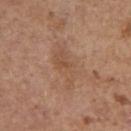Impression:
The lesion was tiled from a total-body skin photograph and was not biopsied.
Clinical summary:
The patient is a female aged approximately 65. Imaged with white-light lighting. The lesion is located on the left upper arm. A lesion tile, about 15 mm wide, cut from a 3D total-body photograph.